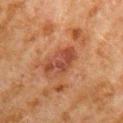Clinical impression: The lesion was photographed on a routine skin check and not biopsied; there is no pathology result. Clinical summary: This image is a 15 mm lesion crop taken from a total-body photograph. The lesion is on the arm. A male subject, aged around 80. The tile uses cross-polarized illumination. Approximately 4.5 mm at its widest.